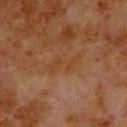No biopsy was performed on this lesion — it was imaged during a full skin examination and was not determined to be concerning. Cropped from a total-body skin-imaging series; the visible field is about 15 mm. A male patient roughly 80 years of age. On the upper back. The lesion's longest dimension is about 4 mm.